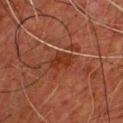<case>
<biopsy_status>not biopsied; imaged during a skin examination</biopsy_status>
<image>
  <source>total-body photography crop</source>
  <field_of_view_mm>15</field_of_view_mm>
</image>
<lesion_size>
  <long_diameter_mm_approx>3.0</long_diameter_mm_approx>
</lesion_size>
<patient>
  <sex>male</sex>
  <age_approx>65</age_approx>
</patient>
<lighting>cross-polarized</lighting>
<site>chest</site>
</case>